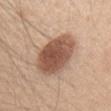This lesion was catalogued during total-body skin photography and was not selected for biopsy.
The lesion's longest dimension is about 6 mm.
A female subject, in their 40s.
An algorithmic analysis of the crop reported an area of roughly 21 mm², an outline eccentricity of about 0.7 (0 = round, 1 = elongated), and two-axis asymmetry of about 0.1. It also reported a border-irregularity rating of about 1.5/10, a color-variation rating of about 4.5/10, and radial color variation of about 1.5. The software also gave a nevus-likeness score of about 95/100.
A lesion tile, about 15 mm wide, cut from a 3D total-body photograph.
This is a white-light tile.
Located on the head or neck.